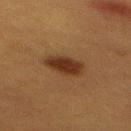Clinical impression:
Part of a total-body skin-imaging series; this lesion was reviewed on a skin check and was not flagged for biopsy.
Context:
About 4 mm across. From the mid back. A roughly 15 mm field-of-view crop from a total-body skin photograph. The subject is a female aged around 40.